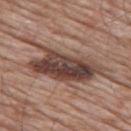Context: The recorded lesion diameter is about 11.5 mm. The lesion-visualizer software estimated a mean CIELAB color near L≈43 a*≈18 b*≈23, roughly 14 lightness units darker than nearby skin, and a normalized border contrast of about 10.5. The software also gave a border-irregularity rating of about 8/10. The analysis additionally found a nevus-likeness score of about 5/100 and a lesion-detection confidence of about 45/100. A 15 mm close-up extracted from a 3D total-body photography capture. The lesion is on the mid back. A male subject, aged 78 to 82. Imaged with white-light lighting.